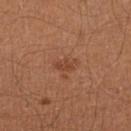Part of a total-body skin-imaging series; this lesion was reviewed on a skin check and was not flagged for biopsy. The tile uses cross-polarized illumination. A lesion tile, about 15 mm wide, cut from a 3D total-body photograph. An algorithmic analysis of the crop reported an average lesion color of about L≈43 a*≈24 b*≈32 (CIELAB), roughly 7 lightness units darker than nearby skin, and a normalized border contrast of about 6. The analysis additionally found a border-irregularity rating of about 4/10, a color-variation rating of about 1.5/10, and peripheral color asymmetry of about 0.5. It also reported a nevus-likeness score of about 0/100 and lesion-presence confidence of about 100/100. A male patient, in their 40s. The recorded lesion diameter is about 2.5 mm. On the left lower leg.